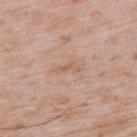Captured during whole-body skin photography for melanoma surveillance; the lesion was not biopsied.
A 15 mm crop from a total-body photograph taken for skin-cancer surveillance.
The patient is a male about 50 years old.
From the upper back.